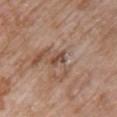follow-up — total-body-photography surveillance lesion; no biopsy
anatomic site — the chest
imaging modality — 15 mm crop, total-body photography
lesion size — ≈2.5 mm
subject — female, approximately 85 years of age or older